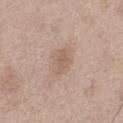Notes:
– notes: catalogued during a skin exam; not biopsied
– subject: male, aged 48 to 52
– automated metrics: a mean CIELAB color near L≈59 a*≈17 b*≈27 and a lesion-to-skin contrast of about 5.5 (normalized; higher = more distinct)
– diameter: ~3 mm (longest diameter)
– anatomic site: the left thigh
– image: ~15 mm crop, total-body skin-cancer survey